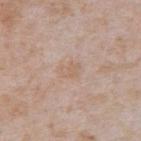Q: Was this lesion biopsied?
A: total-body-photography surveillance lesion; no biopsy
Q: Illumination type?
A: white-light
Q: What are the patient's age and sex?
A: male, in their mid- to late 60s
Q: How large is the lesion?
A: about 2.5 mm
Q: Where on the body is the lesion?
A: the abdomen
Q: What is the imaging modality?
A: ~15 mm crop, total-body skin-cancer survey
Q: Automated lesion metrics?
A: a lesion area of about 4 mm², an eccentricity of roughly 0.75, and two-axis asymmetry of about 0.25; an average lesion color of about L≈62 a*≈16 b*≈28 (CIELAB), about 5 CIELAB-L* units darker than the surrounding skin, and a lesion-to-skin contrast of about 4.5 (normalized; higher = more distinct); a border-irregularity index near 2.5/10, a within-lesion color-variation index near 1.5/10, and radial color variation of about 0.5; a classifier nevus-likeness of about 0/100 and lesion-presence confidence of about 100/100